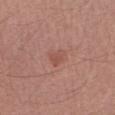Imaged during a routine full-body skin examination; the lesion was not biopsied and no histopathology is available. The recorded lesion diameter is about 2.5 mm. From the right upper arm. Cropped from a whole-body photographic skin survey; the tile spans about 15 mm. This is a white-light tile. The subject is a male aged 53–57. Automated image analysis of the tile measured a lesion area of about 3 mm², an eccentricity of roughly 0.75, and a symmetry-axis asymmetry near 0.3. And it measured a border-irregularity rating of about 3/10, a within-lesion color-variation index near 1/10, and radial color variation of about 0.5.